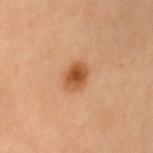The lesion was photographed on a routine skin check and not biopsied; there is no pathology result. On the left upper arm. A 15 mm crop from a total-body photograph taken for skin-cancer surveillance. Automated image analysis of the tile measured a footprint of about 6 mm² and a shape-asymmetry score of about 0.15 (0 = symmetric). The software also gave a border-irregularity rating of about 1/10, a color-variation rating of about 4/10, and a peripheral color-asymmetry measure near 1. It also reported lesion-presence confidence of about 100/100. This is a cross-polarized tile. A female subject aged approximately 55. About 3 mm across.